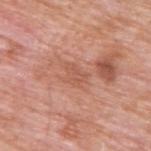Notes:
- notes: total-body-photography surveillance lesion; no biopsy
- anatomic site: the upper back
- illumination: white-light
- lesion diameter: ≈2.5 mm
- acquisition: ~15 mm tile from a whole-body skin photo
- subject: male, approximately 60 years of age